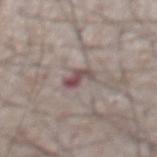<tbp_lesion>
<biopsy_status>not biopsied; imaged during a skin examination</biopsy_status>
<image>
  <source>total-body photography crop</source>
  <field_of_view_mm>15</field_of_view_mm>
</image>
<automated_metrics>
  <border_irregularity_0_10>4.5</border_irregularity_0_10>
  <color_variation_0_10>3.0</color_variation_0_10>
  <peripheral_color_asymmetry>0.5</peripheral_color_asymmetry>
</automated_metrics>
<lesion_size>
  <long_diameter_mm_approx>3.0</long_diameter_mm_approx>
</lesion_size>
<site>abdomen</site>
<lighting>white-light</lighting>
<patient>
  <sex>male</sex>
  <age_approx>75</age_approx>
</patient>
</tbp_lesion>